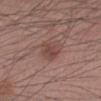No biopsy was performed on this lesion — it was imaged during a full skin examination and was not determined to be concerning. A male patient, aged around 45. The lesion is on the left forearm. Approximately 3.5 mm at its widest. This is a white-light tile. A 15 mm close-up extracted from a 3D total-body photography capture.